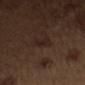<tbp_lesion>
  <biopsy_status>not biopsied; imaged during a skin examination</biopsy_status>
  <lesion_size>
    <long_diameter_mm_approx>2.5</long_diameter_mm_approx>
  </lesion_size>
  <image>
    <source>total-body photography crop</source>
    <field_of_view_mm>15</field_of_view_mm>
  </image>
  <automated_metrics>
    <border_irregularity_0_10>3.5</border_irregularity_0_10>
    <color_variation_0_10>1.5</color_variation_0_10>
    <peripheral_color_asymmetry>0.5</peripheral_color_asymmetry>
  </automated_metrics>
  <lighting>white-light</lighting>
  <site>right upper arm</site>
  <patient>
    <sex>male</sex>
    <age_approx>70</age_approx>
  </patient>
</tbp_lesion>